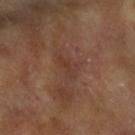Assessment: Imaged during a routine full-body skin examination; the lesion was not biopsied and no histopathology is available. Background: Located on the left forearm. A female patient approximately 75 years of age. A 15 mm crop from a total-body photograph taken for skin-cancer surveillance.